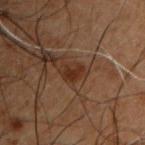Context:
Longest diameter approximately 3 mm. A roughly 15 mm field-of-view crop from a total-body skin photograph. The lesion is on the chest. Automated image analysis of the tile measured a mean CIELAB color near L≈22 a*≈16 b*≈23, roughly 7 lightness units darker than nearby skin, and a normalized border contrast of about 8.5. The software also gave a border-irregularity rating of about 4/10, internal color variation of about 1.5 on a 0–10 scale, and radial color variation of about 0.5. The analysis additionally found a nevus-likeness score of about 50/100. A male subject aged 48 to 52.